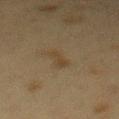Part of a total-body skin-imaging series; this lesion was reviewed on a skin check and was not flagged for biopsy.
This image is a 15 mm lesion crop taken from a total-body photograph.
Measured at roughly 3 mm in maximum diameter.
The total-body-photography lesion software estimated a lesion area of about 3.5 mm² and an eccentricity of roughly 0.9. The analysis additionally found a border-irregularity index near 3.5/10, a color-variation rating of about 0.5/10, and a peripheral color-asymmetry measure near 0. And it measured lesion-presence confidence of about 100/100.
From the mid back.
The tile uses cross-polarized illumination.
The subject is a female aged 38 to 42.